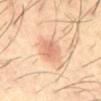Clinical impression: The lesion was tiled from a total-body skin photograph and was not biopsied. Image and clinical context: The lesion-visualizer software estimated an average lesion color of about L≈67 a*≈24 b*≈34 (CIELAB), roughly 10 lightness units darker than nearby skin, and a normalized border contrast of about 6. And it measured a border-irregularity index near 1.5/10, a color-variation rating of about 1.5/10, and peripheral color asymmetry of about 0.5. The analysis additionally found a nevus-likeness score of about 70/100 and a detector confidence of about 100 out of 100 that the crop contains a lesion. This image is a 15 mm lesion crop taken from a total-body photograph. A male subject, approximately 40 years of age. This is a cross-polarized tile. Located on the abdomen.